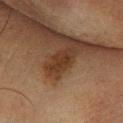notes=total-body-photography surveillance lesion; no biopsy
lesion diameter=≈3 mm
subject=male, in their mid- to late 70s
imaging modality=~15 mm tile from a whole-body skin photo
body site=the left lower leg